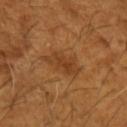{
  "biopsy_status": "not biopsied; imaged during a skin examination",
  "site": "left forearm",
  "image": {
    "source": "total-body photography crop",
    "field_of_view_mm": 15
  },
  "patient": {
    "sex": "male",
    "age_approx": 65
  }
}